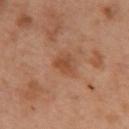Assessment:
This lesion was catalogued during total-body skin photography and was not selected for biopsy.
Acquisition and patient details:
Located on the upper back. A female subject aged 53–57. This is a cross-polarized tile. Cropped from a total-body skin-imaging series; the visible field is about 15 mm.